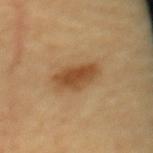Q: Is there a histopathology result?
A: imaged on a skin check; not biopsied
Q: Who is the patient?
A: female, in their mid-40s
Q: Lesion size?
A: ≈4.5 mm
Q: Where on the body is the lesion?
A: the mid back
Q: What kind of image is this?
A: total-body-photography crop, ~15 mm field of view